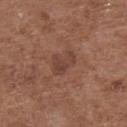• tile lighting · white-light
• image source · total-body-photography crop, ~15 mm field of view
• TBP lesion metrics · a border-irregularity rating of about 4.5/10 and a within-lesion color-variation index near 3/10; a classifier nevus-likeness of about 0/100
• patient · female, in their mid- to late 70s
• lesion diameter · ≈3.5 mm
• anatomic site · the back